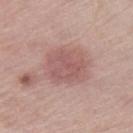<record>
  <biopsy_status>not biopsied; imaged during a skin examination</biopsy_status>
  <lighting>white-light</lighting>
  <image>
    <source>total-body photography crop</source>
    <field_of_view_mm>15</field_of_view_mm>
  </image>
  <patient>
    <sex>female</sex>
    <age_approx>60</age_approx>
  </patient>
  <automated_metrics>
    <nevus_likeness_0_100>5</nevus_likeness_0_100>
    <lesion_detection_confidence_0_100>100</lesion_detection_confidence_0_100>
  </automated_metrics>
  <lesion_size>
    <long_diameter_mm_approx>4.5</long_diameter_mm_approx>
  </lesion_size>
  <site>right thigh</site>
</record>